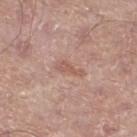<lesion>
<automated_metrics>
  <area_mm2_approx>3.0</area_mm2_approx>
  <eccentricity>0.95</eccentricity>
  <shape_asymmetry>0.45</shape_asymmetry>
  <border_irregularity_0_10>5.0</border_irregularity_0_10>
  <color_variation_0_10>0.0</color_variation_0_10>
  <peripheral_color_asymmetry>0.0</peripheral_color_asymmetry>
  <nevus_likeness_0_100>0</nevus_likeness_0_100>
  <lesion_detection_confidence_0_100>100</lesion_detection_confidence_0_100>
</automated_metrics>
<lesion_size>
  <long_diameter_mm_approx>3.0</long_diameter_mm_approx>
</lesion_size>
<patient>
  <sex>male</sex>
  <age_approx>65</age_approx>
</patient>
<lighting>white-light</lighting>
<image>
  <source>total-body photography crop</source>
  <field_of_view_mm>15</field_of_view_mm>
</image>
<site>left lower leg</site>
</lesion>